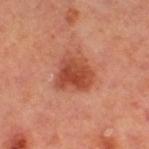Case summary:
* workup — total-body-photography surveillance lesion; no biopsy
* illumination — cross-polarized illumination
* image source — ~15 mm tile from a whole-body skin photo
* lesion size — ≈4 mm
* patient — female
* site — the right thigh